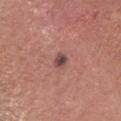Clinical impression:
This lesion was catalogued during total-body skin photography and was not selected for biopsy.
Acquisition and patient details:
From the head or neck. The subject is a female aged 33–37. A 15 mm crop from a total-body photograph taken for skin-cancer surveillance.